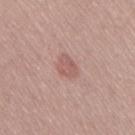Q: Was a biopsy performed?
A: imaged on a skin check; not biopsied
Q: What is the imaging modality?
A: 15 mm crop, total-body photography
Q: What are the patient's age and sex?
A: male, about 70 years old
Q: What is the anatomic site?
A: the lower back
Q: What is the lesion's diameter?
A: ≈3 mm
Q: Illumination type?
A: white-light illumination